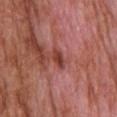<tbp_lesion>
<biopsy_status>not biopsied; imaged during a skin examination</biopsy_status>
<image>
  <source>total-body photography crop</source>
  <field_of_view_mm>15</field_of_view_mm>
</image>
<automated_metrics>
  <vs_skin_darker_L>10.0</vs_skin_darker_L>
  <lesion_detection_confidence_0_100>100</lesion_detection_confidence_0_100>
</automated_metrics>
<patient>
  <sex>male</sex>
  <age_approx>65</age_approx>
</patient>
<lesion_size>
  <long_diameter_mm_approx>2.5</long_diameter_mm_approx>
</lesion_size>
<site>upper back</site>
<lighting>white-light</lighting>
</tbp_lesion>